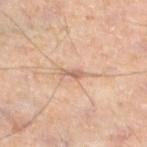The lesion was tiled from a total-body skin photograph and was not biopsied. A male subject, aged 43 to 47. The lesion's longest dimension is about 3 mm. On the leg. A lesion tile, about 15 mm wide, cut from a 3D total-body photograph.